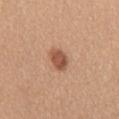Imaged with white-light lighting.
Automated image analysis of the tile measured an area of roughly 5 mm², an outline eccentricity of about 0.75 (0 = round, 1 = elongated), and a symmetry-axis asymmetry near 0.15. And it measured an average lesion color of about L≈53 a*≈23 b*≈32 (CIELAB) and a lesion–skin lightness drop of about 13. The analysis additionally found a nevus-likeness score of about 95/100 and a lesion-detection confidence of about 100/100.
A lesion tile, about 15 mm wide, cut from a 3D total-body photograph.
The patient is a female aged around 55.
The lesion's longest dimension is about 3 mm.
On the mid back.